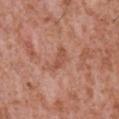Q: Was a biopsy performed?
A: catalogued during a skin exam; not biopsied
Q: What lighting was used for the tile?
A: white-light illumination
Q: How large is the lesion?
A: about 3.5 mm
Q: Patient demographics?
A: male, roughly 45 years of age
Q: How was this image acquired?
A: ~15 mm crop, total-body skin-cancer survey
Q: Lesion location?
A: the chest